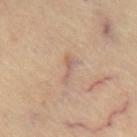biopsy status=catalogued during a skin exam; not biopsied
lighting=cross-polarized
image=~15 mm crop, total-body skin-cancer survey
site=the chest
diameter=about 3.5 mm